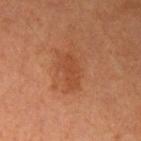Assessment:
The lesion was photographed on a routine skin check and not biopsied; there is no pathology result.
Background:
An algorithmic analysis of the crop reported an automated nevus-likeness rating near 5 out of 100 and lesion-presence confidence of about 100/100. A close-up tile cropped from a whole-body skin photograph, about 15 mm across. The lesion is located on the left upper arm. Approximately 4 mm at its widest. A female patient aged 58–62. The tile uses cross-polarized illumination.